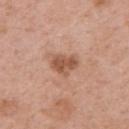About 3.5 mm across.
The tile uses white-light illumination.
A female patient aged approximately 40.
Cropped from a whole-body photographic skin survey; the tile spans about 15 mm.
On the arm.